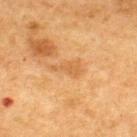The lesion was tiled from a total-body skin photograph and was not biopsied. Cropped from a total-body skin-imaging series; the visible field is about 15 mm. The tile uses cross-polarized illumination. The patient is a male in their mid- to late 70s. Approximately 3.5 mm at its widest. Located on the upper back.